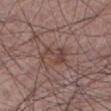Clinical impression: Recorded during total-body skin imaging; not selected for excision or biopsy. Acquisition and patient details: The lesion's longest dimension is about 4 mm. The lesion is on the left lower leg. A male subject, roughly 60 years of age. The tile uses white-light illumination. A 15 mm crop from a total-body photograph taken for skin-cancer surveillance. Automated image analysis of the tile measured a footprint of about 5.5 mm², an outline eccentricity of about 0.85 (0 = round, 1 = elongated), and a symmetry-axis asymmetry near 0.6. And it measured a classifier nevus-likeness of about 0/100 and lesion-presence confidence of about 95/100.